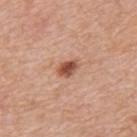Captured during whole-body skin photography for melanoma surveillance; the lesion was not biopsied. A male patient aged 58–62. A region of skin cropped from a whole-body photographic capture, roughly 15 mm wide. Located on the mid back.